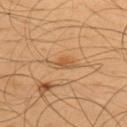Imaged during a routine full-body skin examination; the lesion was not biopsied and no histopathology is available.
A male patient, in their mid-50s.
The lesion is located on the back.
The lesion's longest dimension is about 3.5 mm.
Cropped from a total-body skin-imaging series; the visible field is about 15 mm.
This is a cross-polarized tile.